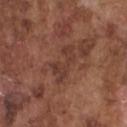{"biopsy_status": "not biopsied; imaged during a skin examination", "lesion_size": {"long_diameter_mm_approx": 5.0}, "lighting": "white-light", "image": {"source": "total-body photography crop", "field_of_view_mm": 15}, "automated_metrics": {"area_mm2_approx": 7.5, "shape_asymmetry": 0.55, "cielab_L": 38, "cielab_a": 21, "cielab_b": 25, "vs_skin_contrast_norm": 6.5}, "site": "chest", "patient": {"sex": "male", "age_approx": 75}}